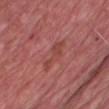biopsy status=imaged on a skin check; not biopsied
acquisition=~15 mm tile from a whole-body skin photo
anatomic site=the chest
patient=male, aged approximately 65